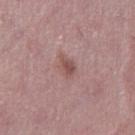| key | value |
|---|---|
| biopsy status | catalogued during a skin exam; not biopsied |
| site | the left thigh |
| patient | female, aged 43–47 |
| imaging modality | ~15 mm tile from a whole-body skin photo |